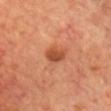The lesion was tiled from a total-body skin photograph and was not biopsied. A male patient aged 68 to 72. The lesion is located on the front of the torso. A region of skin cropped from a whole-body photographic capture, roughly 15 mm wide.